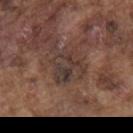Imaged during a routine full-body skin examination; the lesion was not biopsied and no histopathology is available. The recorded lesion diameter is about 4 mm. Captured under white-light illumination. The lesion is located on the mid back. Cropped from a total-body skin-imaging series; the visible field is about 15 mm. A male subject aged 73 to 77.